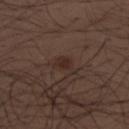Part of a total-body skin-imaging series; this lesion was reviewed on a skin check and was not flagged for biopsy.
On the upper back.
The patient is a male aged approximately 30.
A roughly 15 mm field-of-view crop from a total-body skin photograph.